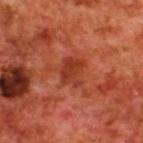This lesion was catalogued during total-body skin photography and was not selected for biopsy. About 3 mm across. The patient is a male aged 68 to 72. From the upper back. A roughly 15 mm field-of-view crop from a total-body skin photograph.